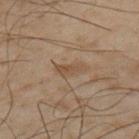Imaged during a routine full-body skin examination; the lesion was not biopsied and no histopathology is available. A male patient roughly 50 years of age. Captured under cross-polarized illumination. On the left upper arm. The lesion's longest dimension is about 3 mm. A lesion tile, about 15 mm wide, cut from a 3D total-body photograph. The lesion-visualizer software estimated an area of roughly 4 mm², a shape eccentricity near 0.85, and a symmetry-axis asymmetry near 0.25. The analysis additionally found a border-irregularity index near 2.5/10 and internal color variation of about 2 on a 0–10 scale.